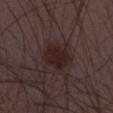notes=no biopsy performed (imaged during a skin exam).